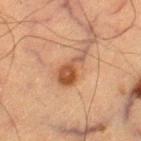biopsy status — total-body-photography surveillance lesion; no biopsy
body site — the right thigh
automated metrics — a lesion color around L≈42 a*≈20 b*≈29 in CIELAB and roughly 10 lightness units darker than nearby skin
image source — total-body-photography crop, ~15 mm field of view
patient — male, in their mid- to late 60s